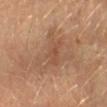biopsy status: total-body-photography surveillance lesion; no biopsy | image: total-body-photography crop, ~15 mm field of view | site: the left forearm | image-analysis metrics: a footprint of about 20 mm², a shape eccentricity near 0.75, and a shape-asymmetry score of about 0.45 (0 = symmetric); an average lesion color of about L≈52 a*≈19 b*≈31 (CIELAB), about 8 CIELAB-L* units darker than the surrounding skin, and a normalized border contrast of about 5.5; a classifier nevus-likeness of about 0/100 and lesion-presence confidence of about 100/100 | subject: male, aged 38–42 | tile lighting: cross-polarized.